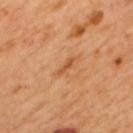| key | value |
|---|---|
| biopsy status | catalogued during a skin exam; not biopsied |
| acquisition | total-body-photography crop, ~15 mm field of view |
| illumination | cross-polarized illumination |
| anatomic site | the back |
| patient | male, aged approximately 50 |
| lesion diameter | ~2.5 mm (longest diameter) |
| image-analysis metrics | a lesion color around L≈54 a*≈26 b*≈41 in CIELAB, about 9 CIELAB-L* units darker than the surrounding skin, and a normalized border contrast of about 6.5; a border-irregularity index near 3.5/10, a within-lesion color-variation index near 0/10, and peripheral color asymmetry of about 0; a nevus-likeness score of about 0/100 and a lesion-detection confidence of about 100/100 |